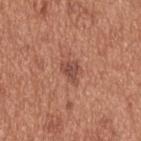Imaged during a routine full-body skin examination; the lesion was not biopsied and no histopathology is available. Automated image analysis of the tile measured a shape eccentricity near 0.65 and two-axis asymmetry of about 0.45. Cropped from a total-body skin-imaging series; the visible field is about 15 mm. The tile uses white-light illumination. A male patient, aged 53–57. Located on the upper back.